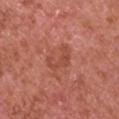No biopsy was performed on this lesion — it was imaged during a full skin examination and was not determined to be concerning.